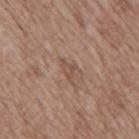The lesion was tiled from a total-body skin photograph and was not biopsied. Located on the mid back. A male subject aged around 70. About 2.5 mm across. The tile uses white-light illumination. Automated image analysis of the tile measured an area of roughly 2.5 mm² and a symmetry-axis asymmetry near 0.45. The analysis additionally found border irregularity of about 4.5 on a 0–10 scale and radial color variation of about 0.5. It also reported an automated nevus-likeness rating near 0 out of 100 and a lesion-detection confidence of about 95/100. A roughly 15 mm field-of-view crop from a total-body skin photograph.